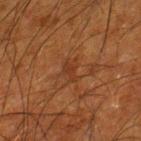  biopsy_status: not biopsied; imaged during a skin examination
  site: right lower leg
  image:
    source: total-body photography crop
    field_of_view_mm: 15
  patient:
    sex: male
    age_approx: 65